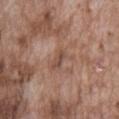The lesion was photographed on a routine skin check and not biopsied; there is no pathology result. The lesion is on the abdomen. Measured at roughly 2.5 mm in maximum diameter. Cropped from a total-body skin-imaging series; the visible field is about 15 mm. The patient is a male aged approximately 75. This is a white-light tile. Automated tile analysis of the lesion measured a lesion area of about 2.5 mm² and a shape-asymmetry score of about 0.35 (0 = symmetric). It also reported about 9 CIELAB-L* units darker than the surrounding skin and a normalized border contrast of about 6.5. The analysis additionally found border irregularity of about 3.5 on a 0–10 scale.